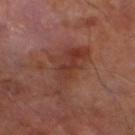<lesion>
  <biopsy_status>not biopsied; imaged during a skin examination</biopsy_status>
  <patient>
    <sex>male</sex>
    <age_approx>70</age_approx>
  </patient>
  <automated_metrics>
    <cielab_L>36</cielab_L>
    <cielab_a>23</cielab_a>
    <cielab_b>26</cielab_b>
    <vs_skin_darker_L>7.0</vs_skin_darker_L>
    <vs_skin_contrast_norm>6.5</vs_skin_contrast_norm>
    <color_variation_0_10>4.0</color_variation_0_10>
    <lesion_detection_confidence_0_100>100</lesion_detection_confidence_0_100>
  </automated_metrics>
  <lighting>cross-polarized</lighting>
  <site>left lower leg</site>
  <image>
    <source>total-body photography crop</source>
    <field_of_view_mm>15</field_of_view_mm>
  </image>
</lesion>